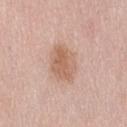Impression:
Imaged during a routine full-body skin examination; the lesion was not biopsied and no histopathology is available.
Context:
A female patient roughly 60 years of age. The lesion is located on the lower back. This is a white-light tile. Approximately 4.5 mm at its widest. A 15 mm close-up tile from a total-body photography series done for melanoma screening. The total-body-photography lesion software estimated a lesion area of about 13 mm², an eccentricity of roughly 0.65, and two-axis asymmetry of about 0.2. The analysis additionally found an average lesion color of about L≈62 a*≈20 b*≈30 (CIELAB) and roughly 9 lightness units darker than nearby skin. The analysis additionally found border irregularity of about 1.5 on a 0–10 scale and radial color variation of about 1.